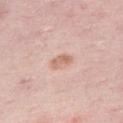Notes:
• workup — imaged on a skin check; not biopsied
• illumination — white-light illumination
• imaging modality — 15 mm crop, total-body photography
• automated metrics — an area of roughly 3.5 mm², an eccentricity of roughly 0.8, and a symmetry-axis asymmetry near 0.3; an average lesion color of about L≈66 a*≈21 b*≈29 (CIELAB), about 9 CIELAB-L* units darker than the surrounding skin, and a normalized border contrast of about 6.5; a nevus-likeness score of about 45/100
• anatomic site — the right thigh
• subject — female, approximately 45 years of age
• diameter — ~2.5 mm (longest diameter)